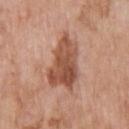Recorded during total-body skin imaging; not selected for excision or biopsy.
Approximately 6.5 mm at its widest.
The subject is a male aged 58 to 62.
Cropped from a whole-body photographic skin survey; the tile spans about 15 mm.
The lesion is on the upper back.
Captured under white-light illumination.